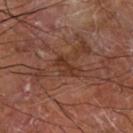Notes:
- workup — imaged on a skin check; not biopsied
- automated lesion analysis — a lesion area of about 5.5 mm² and an eccentricity of roughly 0.8; a border-irregularity rating of about 3.5/10, internal color variation of about 2.5 on a 0–10 scale, and radial color variation of about 1; a lesion-detection confidence of about 95/100
- location — the right forearm
- lesion diameter — ≈3.5 mm
- patient — male, roughly 65 years of age
- image source — ~15 mm crop, total-body skin-cancer survey
- illumination — cross-polarized